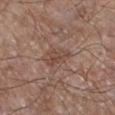biopsy status = catalogued during a skin exam; not biopsied
patient = male, approximately 65 years of age
site = the right lower leg
lesion diameter = about 3.5 mm
tile lighting = white-light
TBP lesion metrics = an eccentricity of roughly 0.85 and two-axis asymmetry of about 0.4
imaging modality = ~15 mm tile from a whole-body skin photo